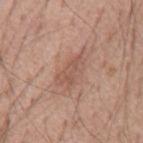Impression: This lesion was catalogued during total-body skin photography and was not selected for biopsy. Acquisition and patient details: A roughly 15 mm field-of-view crop from a total-body skin photograph. The recorded lesion diameter is about 5 mm. A male subject, approximately 55 years of age. The lesion is on the mid back.